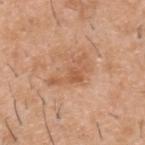The lesion was photographed on a routine skin check and not biopsied; there is no pathology result.
A lesion tile, about 15 mm wide, cut from a 3D total-body photograph.
Captured under white-light illumination.
Located on the upper back.
Approximately 5.5 mm at its widest.
A male subject, aged 38 to 42.
Automated tile analysis of the lesion measured an area of roughly 7 mm², a shape eccentricity near 0.9, and a shape-asymmetry score of about 0.6 (0 = symmetric). The analysis additionally found a border-irregularity index near 9.5/10, internal color variation of about 2 on a 0–10 scale, and a peripheral color-asymmetry measure near 0.5. The analysis additionally found a nevus-likeness score of about 0/100.